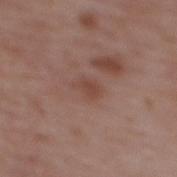Imaged during a routine full-body skin examination; the lesion was not biopsied and no histopathology is available. Located on the upper back. Longest diameter approximately 3 mm. Imaged with white-light lighting. An algorithmic analysis of the crop reported a border-irregularity rating of about 3/10, internal color variation of about 1 on a 0–10 scale, and a peripheral color-asymmetry measure near 0.5. A female patient aged 63–67. A lesion tile, about 15 mm wide, cut from a 3D total-body photograph.